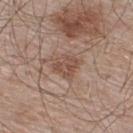The lesion was tiled from a total-body skin photograph and was not biopsied. The tile uses white-light illumination. A male subject, about 55 years old. Located on the back. The total-body-photography lesion software estimated a footprint of about 6.5 mm², an eccentricity of roughly 0.3, and a symmetry-axis asymmetry near 0.2. A region of skin cropped from a whole-body photographic capture, roughly 15 mm wide.